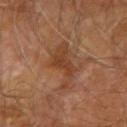Impression:
The lesion was tiled from a total-body skin photograph and was not biopsied.
Background:
Automated tile analysis of the lesion measured a lesion color around L≈41 a*≈22 b*≈32 in CIELAB, a lesion–skin lightness drop of about 8, and a normalized border contrast of about 7. It also reported a lesion-detection confidence of about 100/100. About 6 mm across. On the right upper arm. Cropped from a whole-body photographic skin survey; the tile spans about 15 mm. The patient is a male roughly 70 years of age.